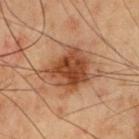| field | value |
|---|---|
| location | the chest |
| acquisition | total-body-photography crop, ~15 mm field of view |
| patient | male, roughly 55 years of age |